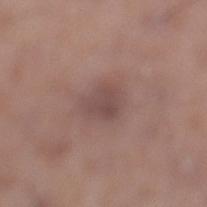Part of a total-body skin-imaging series; this lesion was reviewed on a skin check and was not flagged for biopsy.
This image is a 15 mm lesion crop taken from a total-body photograph.
The subject is a male aged 53 to 57.
On the left lower leg.